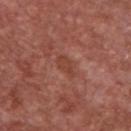Q: Is there a histopathology result?
A: catalogued during a skin exam; not biopsied
Q: Illumination type?
A: white-light
Q: What is the lesion's diameter?
A: ~3 mm (longest diameter)
Q: What did automated image analysis measure?
A: an area of roughly 4 mm² and a shape eccentricity near 0.9; a border-irregularity index near 3/10, a within-lesion color-variation index near 1/10, and peripheral color asymmetry of about 0.5
Q: How was this image acquired?
A: ~15 mm crop, total-body skin-cancer survey
Q: Lesion location?
A: the front of the torso
Q: Patient demographics?
A: male, aged 63–67